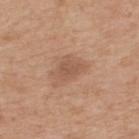Captured during whole-body skin photography for melanoma surveillance; the lesion was not biopsied. This image is a 15 mm lesion crop taken from a total-body photograph. Located on the back. Imaged with white-light lighting. Automated image analysis of the tile measured an area of roughly 5.5 mm², a shape eccentricity near 0.6, and a shape-asymmetry score of about 0.35 (0 = symmetric). It also reported a mean CIELAB color near L≈54 a*≈20 b*≈30, a lesion–skin lightness drop of about 8, and a normalized lesion–skin contrast near 5.5. The software also gave a border-irregularity index near 3.5/10 and a color-variation rating of about 1.5/10. A male subject, in their mid- to late 60s.